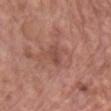Notes:
- notes: imaged on a skin check; not biopsied
- body site: the chest
- image: 15 mm crop, total-body photography
- illumination: white-light
- subject: male, aged approximately 80
- image-analysis metrics: an area of roughly 3 mm², an outline eccentricity of about 0.85 (0 = round, 1 = elongated), and two-axis asymmetry of about 0.3; a border-irregularity rating of about 2.5/10 and a within-lesion color-variation index near 1/10; a nevus-likeness score of about 0/100